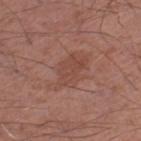Q: Was this lesion biopsied?
A: catalogued during a skin exam; not biopsied
Q: What is the lesion's diameter?
A: ≈5.5 mm
Q: Illumination type?
A: white-light illumination
Q: What did automated image analysis measure?
A: a mean CIELAB color near L≈47 a*≈22 b*≈26, roughly 6 lightness units darker than nearby skin, and a normalized lesion–skin contrast near 5; a classifier nevus-likeness of about 0/100
Q: Patient demographics?
A: male, aged 53–57
Q: How was this image acquired?
A: 15 mm crop, total-body photography
Q: Lesion location?
A: the left thigh